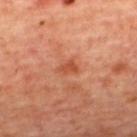<record>
  <site>upper back</site>
  <patient>
    <sex>female</sex>
    <age_approx>45</age_approx>
  </patient>
  <image>
    <source>total-body photography crop</source>
    <field_of_view_mm>15</field_of_view_mm>
  </image>
</record>